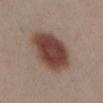Impression:
The lesion was photographed on a routine skin check and not biopsied; there is no pathology result.
Acquisition and patient details:
On the abdomen. The recorded lesion diameter is about 6.5 mm. The patient is a female roughly 30 years of age. A 15 mm close-up extracted from a 3D total-body photography capture. This is a white-light tile. An algorithmic analysis of the crop reported a lesion area of about 24 mm², a shape eccentricity near 0.7, and a shape-asymmetry score of about 0.15 (0 = symmetric). It also reported a lesion color around L≈43 a*≈20 b*≈24 in CIELAB, roughly 15 lightness units darker than nearby skin, and a normalized lesion–skin contrast near 11.5. It also reported border irregularity of about 1.5 on a 0–10 scale, internal color variation of about 4.5 on a 0–10 scale, and a peripheral color-asymmetry measure near 1.